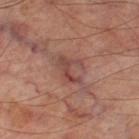<lesion>
<lesion_size>
  <long_diameter_mm_approx>3.5</long_diameter_mm_approx>
</lesion_size>
<site>left thigh</site>
<image>
  <source>total-body photography crop</source>
  <field_of_view_mm>15</field_of_view_mm>
</image>
<patient>
  <sex>male</sex>
  <age_approx>70</age_approx>
</patient>
<automated_metrics>
  <area_mm2_approx>5.5</area_mm2_approx>
  <eccentricity>0.7</eccentricity>
  <shape_asymmetry>0.6</shape_asymmetry>
</automated_metrics>
</lesion>